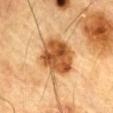Q: Is there a histopathology result?
A: no biopsy performed (imaged during a skin exam)
Q: Who is the patient?
A: male, roughly 85 years of age or older
Q: Lesion size?
A: about 5.5 mm
Q: How was the tile lit?
A: cross-polarized
Q: What did automated image analysis measure?
A: a lesion area of about 19 mm², an outline eccentricity of about 0.45 (0 = round, 1 = elongated), and a symmetry-axis asymmetry near 0.15
Q: Where on the body is the lesion?
A: the chest
Q: What is the imaging modality?
A: ~15 mm crop, total-body skin-cancer survey